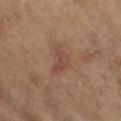Assessment:
Imaged during a routine full-body skin examination; the lesion was not biopsied and no histopathology is available.
Acquisition and patient details:
This image is a 15 mm lesion crop taken from a total-body photograph. Imaged with cross-polarized lighting. A female subject, approximately 75 years of age. The recorded lesion diameter is about 4 mm. Located on the left lower leg.